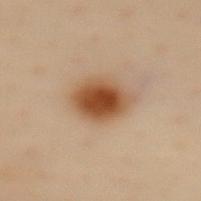• workup — no biopsy performed (imaged during a skin exam)
• image source — 15 mm crop, total-body photography
• lesion size — about 4.5 mm
• location — the mid back
• patient — female, aged around 60
• tile lighting — cross-polarized